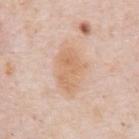Clinical impression: The lesion was tiled from a total-body skin photograph and was not biopsied. Background: A male subject aged around 80. Captured under white-light illumination. On the chest. Automated image analysis of the tile measured internal color variation of about 2.5 on a 0–10 scale and a peripheral color-asymmetry measure near 1. A region of skin cropped from a whole-body photographic capture, roughly 15 mm wide. About 5 mm across.